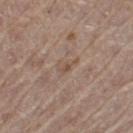Imaged during a routine full-body skin examination; the lesion was not biopsied and no histopathology is available.
A lesion tile, about 15 mm wide, cut from a 3D total-body photograph.
The lesion-visualizer software estimated a lesion area of about 2.5 mm², an outline eccentricity of about 0.9 (0 = round, 1 = elongated), and a symmetry-axis asymmetry near 0.35. And it measured a lesion–skin lightness drop of about 7. And it measured border irregularity of about 3.5 on a 0–10 scale, a color-variation rating of about 1/10, and peripheral color asymmetry of about 0. And it measured a nevus-likeness score of about 0/100.
The lesion is on the left thigh.
A male subject about 65 years old.